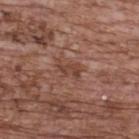Captured during whole-body skin photography for melanoma surveillance; the lesion was not biopsied. This image is a 15 mm lesion crop taken from a total-body photograph. The lesion's longest dimension is about 3 mm. Automated image analysis of the tile measured a footprint of about 4 mm², an outline eccentricity of about 0.85 (0 = round, 1 = elongated), and two-axis asymmetry of about 0.4. And it measured a border-irregularity index near 4.5/10, a within-lesion color-variation index near 2/10, and a peripheral color-asymmetry measure near 0.5. The analysis additionally found a nevus-likeness score of about 0/100 and a detector confidence of about 100 out of 100 that the crop contains a lesion. A female subject in their mid-60s. Captured under white-light illumination. From the upper back.